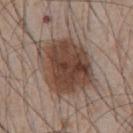biopsy_status: not biopsied; imaged during a skin examination
lighting: white-light
patient:
  sex: male
  age_approx: 45
image:
  source: total-body photography crop
  field_of_view_mm: 15
lesion_size:
  long_diameter_mm_approx: 6.5
site: front of the torso